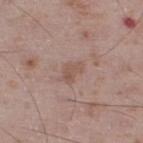This lesion was catalogued during total-body skin photography and was not selected for biopsy.
A male patient, about 75 years old.
Captured under white-light illumination.
The lesion is located on the left lower leg.
A lesion tile, about 15 mm wide, cut from a 3D total-body photograph.
The recorded lesion diameter is about 3 mm.